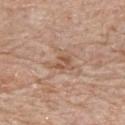Captured during whole-body skin photography for melanoma surveillance; the lesion was not biopsied. A region of skin cropped from a whole-body photographic capture, roughly 15 mm wide. This is a white-light tile. The lesion is located on the upper back. The recorded lesion diameter is about 3.5 mm. The lesion-visualizer software estimated a lesion color around L≈55 a*≈19 b*≈30 in CIELAB, roughly 8 lightness units darker than nearby skin, and a normalized lesion–skin contrast near 6. The software also gave a classifier nevus-likeness of about 0/100 and a lesion-detection confidence of about 100/100. The subject is a female approximately 85 years of age or older.